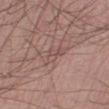A male subject, in their mid-50s. Cropped from a total-body skin-imaging series; the visible field is about 15 mm. Automated image analysis of the tile measured a lesion area of about 3.5 mm², an eccentricity of roughly 0.8, and two-axis asymmetry of about 0.45. And it measured an average lesion color of about L≈51 a*≈19 b*≈22 (CIELAB), roughly 6 lightness units darker than nearby skin, and a normalized border contrast of about 4.5. The analysis additionally found peripheral color asymmetry of about 1. Approximately 2.5 mm at its widest. From the right lower leg. Imaged with white-light lighting.